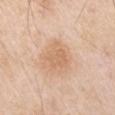{"biopsy_status": "not biopsied; imaged during a skin examination", "patient": {"sex": "male", "age_approx": 65}, "image": {"source": "total-body photography crop", "field_of_view_mm": 15}, "lighting": "white-light", "site": "left upper arm", "lesion_size": {"long_diameter_mm_approx": 3.5}}